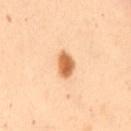This lesion was catalogued during total-body skin photography and was not selected for biopsy.
The tile uses cross-polarized illumination.
A roughly 15 mm field-of-view crop from a total-body skin photograph.
The total-body-photography lesion software estimated a lesion color around L≈56 a*≈22 b*≈37 in CIELAB, a lesion–skin lightness drop of about 15, and a normalized lesion–skin contrast near 10.5.
A female patient roughly 50 years of age.
On the mid back.
The recorded lesion diameter is about 3.5 mm.